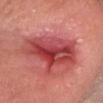  biopsy_status: not biopsied; imaged during a skin examination
  lighting: white-light
  image:
    source: total-body photography crop
    field_of_view_mm: 15
  patient:
    sex: female
    age_approx: 50
  lesion_size:
    long_diameter_mm_approx: 8.5
  site: head or neck